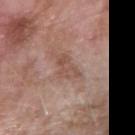| feature | finding |
|---|---|
| follow-up | total-body-photography surveillance lesion; no biopsy |
| patient | female, about 75 years old |
| automated lesion analysis | an area of roughly 5.5 mm², a shape eccentricity near 0.85, and a shape-asymmetry score of about 0.4 (0 = symmetric); a mean CIELAB color near L≈51 a*≈19 b*≈25, a lesion–skin lightness drop of about 8, and a lesion-to-skin contrast of about 6 (normalized; higher = more distinct); a lesion-detection confidence of about 95/100 |
| image | total-body-photography crop, ~15 mm field of view |
| body site | the right forearm |
| lesion size | ~4 mm (longest diameter) |